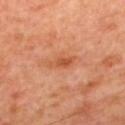This lesion was catalogued during total-body skin photography and was not selected for biopsy.
The lesion-visualizer software estimated a symmetry-axis asymmetry near 0.3. The analysis additionally found a mean CIELAB color near L≈53 a*≈29 b*≈39 and a lesion–skin lightness drop of about 8. And it measured a classifier nevus-likeness of about 0/100 and a lesion-detection confidence of about 100/100.
About 3 mm across.
On the upper back.
This image is a 15 mm lesion crop taken from a total-body photograph.
Imaged with cross-polarized lighting.
The subject is a male approximately 60 years of age.